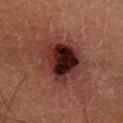| field | value |
|---|---|
| image-analysis metrics | a footprint of about 24 mm², an outline eccentricity of about 0.65 (0 = round, 1 = elongated), and two-axis asymmetry of about 0.2 |
| image source | 15 mm crop, total-body photography |
| body site | the right forearm |
| illumination | cross-polarized illumination |
| lesion diameter | ≈6.5 mm |
| subject | male, aged around 60 |